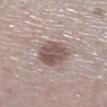follow-up — catalogued during a skin exam; not biopsied | tile lighting — white-light illumination | body site — the right lower leg | acquisition — ~15 mm crop, total-body skin-cancer survey | lesion size — about 4 mm | patient — male, approximately 60 years of age.